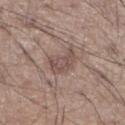Recorded during total-body skin imaging; not selected for excision or biopsy. The lesion's longest dimension is about 3.5 mm. An algorithmic analysis of the crop reported a footprint of about 7 mm² and two-axis asymmetry of about 0.45. And it measured a mean CIELAB color near L≈50 a*≈16 b*≈21 and a normalized lesion–skin contrast near 6.5. The analysis additionally found border irregularity of about 4.5 on a 0–10 scale, a within-lesion color-variation index near 2.5/10, and radial color variation of about 1. Located on the right lower leg. A male subject, aged approximately 60. A lesion tile, about 15 mm wide, cut from a 3D total-body photograph. Imaged with white-light lighting.